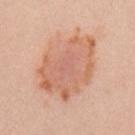The lesion was photographed on a routine skin check and not biopsied; there is no pathology result. A lesion tile, about 15 mm wide, cut from a 3D total-body photograph. Measured at roughly 8.5 mm in maximum diameter. This is a white-light tile. Automated image analysis of the tile measured an area of roughly 41 mm², a shape eccentricity near 0.65, and a shape-asymmetry score of about 0.2 (0 = symmetric). The analysis additionally found an automated nevus-likeness rating near 10 out of 100 and lesion-presence confidence of about 100/100. On the left upper arm. A female subject in their mid- to late 20s.